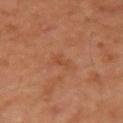Part of a total-body skin-imaging series; this lesion was reviewed on a skin check and was not flagged for biopsy. The patient is a female aged approximately 55. The total-body-photography lesion software estimated an average lesion color of about L≈50 a*≈26 b*≈36 (CIELAB), about 6 CIELAB-L* units darker than the surrounding skin, and a normalized lesion–skin contrast near 4.5. The analysis additionally found a color-variation rating of about 0/10 and peripheral color asymmetry of about 0. A region of skin cropped from a whole-body photographic capture, roughly 15 mm wide. This is a cross-polarized tile. The lesion's longest dimension is about 2.5 mm. Located on the arm.